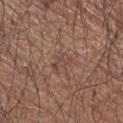Captured during whole-body skin photography for melanoma surveillance; the lesion was not biopsied.
This image is a 15 mm lesion crop taken from a total-body photograph.
The subject is a male in their mid-60s.
From the right upper arm.